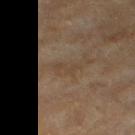Part of a total-body skin-imaging series; this lesion was reviewed on a skin check and was not flagged for biopsy. On the right thigh. A female subject, aged 58 to 62. This image is a 15 mm lesion crop taken from a total-body photograph.